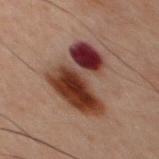Q: Is there a histopathology result?
A: catalogued during a skin exam; not biopsied
Q: What is the lesion's diameter?
A: ≈8 mm
Q: Patient demographics?
A: male, roughly 60 years of age
Q: What kind of image is this?
A: total-body-photography crop, ~15 mm field of view
Q: What is the anatomic site?
A: the front of the torso
Q: What lighting was used for the tile?
A: cross-polarized